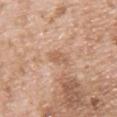Part of a total-body skin-imaging series; this lesion was reviewed on a skin check and was not flagged for biopsy.
Approximately 2.5 mm at its widest.
A male patient aged around 50.
The lesion is located on the chest.
Cropped from a total-body skin-imaging series; the visible field is about 15 mm.